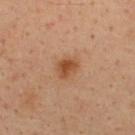workup: total-body-photography surveillance lesion; no biopsy
automated metrics: an area of roughly 6 mm² and two-axis asymmetry of about 0.2; a mean CIELAB color near L≈46 a*≈21 b*≈33 and a lesion–skin lightness drop of about 9; border irregularity of about 2 on a 0–10 scale and a peripheral color-asymmetry measure near 1
patient: male, aged 33 to 37
acquisition: ~15 mm tile from a whole-body skin photo
anatomic site: the upper back